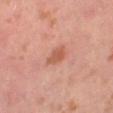follow-up: total-body-photography surveillance lesion; no biopsy | tile lighting: cross-polarized | location: the left lower leg | patient: female, roughly 40 years of age | automated lesion analysis: a footprint of about 4 mm², an eccentricity of roughly 0.55, and a shape-asymmetry score of about 0.25 (0 = symmetric); roughly 9 lightness units darker than nearby skin and a normalized border contrast of about 6.5; a border-irregularity index near 2.5/10, a within-lesion color-variation index near 2/10, and a peripheral color-asymmetry measure near 0.5 | image: 15 mm crop, total-body photography.